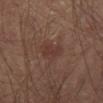Q: Was a biopsy performed?
A: catalogued during a skin exam; not biopsied
Q: What did automated image analysis measure?
A: a lesion area of about 5.5 mm², an outline eccentricity of about 0.65 (0 = round, 1 = elongated), and two-axis asymmetry of about 0.3; a border-irregularity rating of about 3/10, a within-lesion color-variation index near 1.5/10, and radial color variation of about 0.5
Q: How large is the lesion?
A: about 3 mm
Q: Patient demographics?
A: male, aged 63 to 67
Q: What is the imaging modality?
A: ~15 mm crop, total-body skin-cancer survey
Q: What is the anatomic site?
A: the right thigh
Q: What lighting was used for the tile?
A: cross-polarized